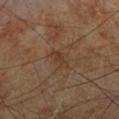Case summary:
• notes — imaged on a skin check; not biopsied
• diameter — about 3.5 mm
• illumination — cross-polarized
• subject — male, roughly 70 years of age
• automated lesion analysis — a lesion color around L≈36 a*≈17 b*≈28 in CIELAB and a normalized border contrast of about 5.5; an automated nevus-likeness rating near 0 out of 100 and a lesion-detection confidence of about 100/100
• acquisition — total-body-photography crop, ~15 mm field of view
• location — the right lower leg